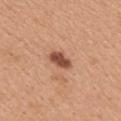Q: Illumination type?
A: white-light illumination
Q: What kind of image is this?
A: 15 mm crop, total-body photography
Q: Patient demographics?
A: female, in their 30s
Q: Where on the body is the lesion?
A: the upper back
Q: How large is the lesion?
A: ~3 mm (longest diameter)
Q: What did automated image analysis measure?
A: a mean CIELAB color near L≈50 a*≈26 b*≈31, a lesion–skin lightness drop of about 16, and a normalized lesion–skin contrast near 10.5; a border-irregularity rating of about 2.5/10; an automated nevus-likeness rating near 90 out of 100 and lesion-presence confidence of about 100/100